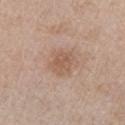{
  "site": "right upper arm",
  "patient": {
    "sex": "female",
    "age_approx": 65
  },
  "lighting": "white-light",
  "lesion_size": {
    "long_diameter_mm_approx": 3.0
  },
  "image": {
    "source": "total-body photography crop",
    "field_of_view_mm": 15
  },
  "automated_metrics": {
    "vs_skin_darker_L": 7.0,
    "vs_skin_contrast_norm": 5.5,
    "nevus_likeness_0_100": 5,
    "lesion_detection_confidence_0_100": 100
  }
}